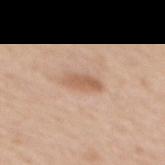biopsy_status: not biopsied; imaged during a skin examination
lesion_size:
  long_diameter_mm_approx: 3.5
image:
  source: total-body photography crop
  field_of_view_mm: 15
lighting: white-light
site: mid back
patient:
  sex: female
  age_approx: 70